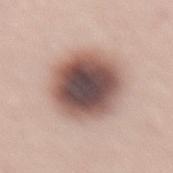The lesion was tiled from a total-body skin photograph and was not biopsied. From the lower back. Approximately 7 mm at its widest. Captured under white-light illumination. The total-body-photography lesion software estimated a footprint of about 32 mm², a shape eccentricity near 0.6, and two-axis asymmetry of about 0.1. The analysis additionally found a lesion–skin lightness drop of about 21 and a lesion-to-skin contrast of about 13 (normalized; higher = more distinct). It also reported a nevus-likeness score of about 25/100 and a lesion-detection confidence of about 100/100. A male subject, aged 53 to 57. A 15 mm crop from a total-body photograph taken for skin-cancer surveillance.